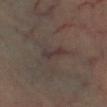The total-body-photography lesion software estimated a border-irregularity index near 4/10 and a color-variation rating of about 0/10. On the right leg. This is a cross-polarized tile. A female patient, roughly 60 years of age. The lesion's longest dimension is about 3 mm. A lesion tile, about 15 mm wide, cut from a 3D total-body photograph.